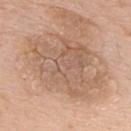{"lesion_size": {"long_diameter_mm_approx": 9.0}, "lighting": "white-light", "patient": {"sex": "male", "age_approx": 60}, "image": {"source": "total-body photography crop", "field_of_view_mm": 15}, "site": "upper back"}